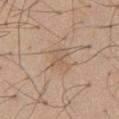notes: no biopsy performed (imaged during a skin exam)
image source: 15 mm crop, total-body photography
automated lesion analysis: a footprint of about 6 mm², an eccentricity of roughly 0.65, and a shape-asymmetry score of about 0.4 (0 = symmetric); an average lesion color of about L≈58 a*≈16 b*≈30 (CIELAB) and a normalized border contrast of about 4.5
body site: the abdomen
subject: male, aged 53 to 57
lesion diameter: ≈3 mm
lighting: white-light illumination